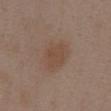Case summary:
* follow-up — catalogued during a skin exam; not biopsied
* illumination — white-light illumination
* subject — female, aged 53–57
* image source — ~15 mm crop, total-body skin-cancer survey
* automated lesion analysis — an outline eccentricity of about 0.55 (0 = round, 1 = elongated) and a shape-asymmetry score of about 0.2 (0 = symmetric); an average lesion color of about L≈47 a*≈16 b*≈26 (CIELAB), a lesion–skin lightness drop of about 6, and a normalized border contrast of about 5.5
* body site — the abdomen
* diameter — ~4 mm (longest diameter)